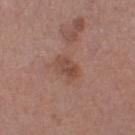Clinical impression:
The lesion was photographed on a routine skin check and not biopsied; there is no pathology result.
Context:
About 3.5 mm across. Located on the right thigh. The total-body-photography lesion software estimated a footprint of about 5.5 mm², an eccentricity of roughly 0.8, and a symmetry-axis asymmetry near 0.15. And it measured a border-irregularity index near 1.5/10, a color-variation rating of about 3.5/10, and peripheral color asymmetry of about 1.5. A male patient in their mid-70s. This image is a 15 mm lesion crop taken from a total-body photograph. The tile uses white-light illumination.